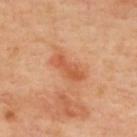The lesion was photographed on a routine skin check and not biopsied; there is no pathology result. A 15 mm close-up extracted from a 3D total-body photography capture. The lesion's longest dimension is about 4.5 mm. An algorithmic analysis of the crop reported a lesion area of about 6.5 mm², an eccentricity of roughly 0.9, and a shape-asymmetry score of about 0.25 (0 = symmetric). The software also gave about 9 CIELAB-L* units darker than the surrounding skin and a normalized lesion–skin contrast near 6.5. The software also gave a border-irregularity index near 3/10, internal color variation of about 4 on a 0–10 scale, and radial color variation of about 1.5. A female patient roughly 40 years of age. From the upper back. Captured under cross-polarized illumination.